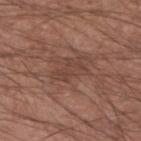| field | value |
|---|---|
| notes | no biopsy performed (imaged during a skin exam) |